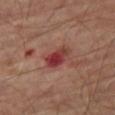biopsy status=total-body-photography surveillance lesion; no biopsy | tile lighting=cross-polarized illumination | subject=male, aged 68 to 72 | body site=the right thigh | lesion size=~3.5 mm (longest diameter) | image source=15 mm crop, total-body photography.